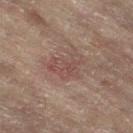The lesion was photographed on a routine skin check and not biopsied; there is no pathology result. The subject is a female roughly 80 years of age. The lesion is located on the left leg. An algorithmic analysis of the crop reported a lesion area of about 9.5 mm² and a shape eccentricity near 0.55. The software also gave an average lesion color of about L≈43 a*≈17 b*≈20 (CIELAB) and a lesion-to-skin contrast of about 5 (normalized; higher = more distinct). Captured under cross-polarized illumination. The recorded lesion diameter is about 4 mm. A roughly 15 mm field-of-view crop from a total-body skin photograph.